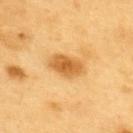biopsy status — imaged on a skin check; not biopsied
subject — male, roughly 60 years of age
site — the upper back
lighting — cross-polarized illumination
image source — 15 mm crop, total-body photography